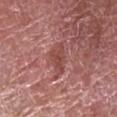Findings:
• workup — imaged on a skin check; not biopsied
• image-analysis metrics — an outline eccentricity of about 0.8 (0 = round, 1 = elongated); a lesion color around L≈47 a*≈27 b*≈24 in CIELAB, a lesion–skin lightness drop of about 8, and a lesion-to-skin contrast of about 6.5 (normalized; higher = more distinct); a border-irregularity index near 4/10, a within-lesion color-variation index near 2/10, and peripheral color asymmetry of about 0.5
• imaging modality — ~15 mm crop, total-body skin-cancer survey
• subject — female, aged 58–62
• anatomic site — the right forearm
• diameter — ≈3.5 mm
• lighting — white-light